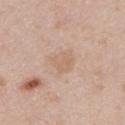| key | value |
|---|---|
| imaging modality | 15 mm crop, total-body photography |
| lesion diameter | about 3 mm |
| tile lighting | white-light illumination |
| patient | male, in their 40s |
| location | the back |